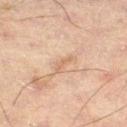Impression:
Recorded during total-body skin imaging; not selected for excision or biopsy.
Image and clinical context:
Captured under cross-polarized illumination. A lesion tile, about 15 mm wide, cut from a 3D total-body photograph. Measured at roughly 2.5 mm in maximum diameter. The lesion is located on the leg. A male subject approximately 60 years of age. The lesion-visualizer software estimated a footprint of about 2 mm². And it measured an average lesion color of about L≈48 a*≈15 b*≈26 (CIELAB) and a lesion-to-skin contrast of about 5 (normalized; higher = more distinct).